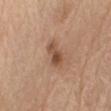{"biopsy_status": "not biopsied; imaged during a skin examination", "site": "right upper arm", "lesion_size": {"long_diameter_mm_approx": 3.5}, "automated_metrics": {"border_irregularity_0_10": 3.0, "color_variation_0_10": 3.5, "peripheral_color_asymmetry": 1.0, "lesion_detection_confidence_0_100": 100}, "image": {"source": "total-body photography crop", "field_of_view_mm": 15}, "patient": {"sex": "male", "age_approx": 70}}